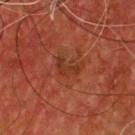biopsy_status: not biopsied; imaged during a skin examination
patient:
  sex: male
  age_approx: 55
site: chest
image:
  source: total-body photography crop
  field_of_view_mm: 15